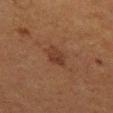biopsy status — total-body-photography surveillance lesion; no biopsy
imaging modality — ~15 mm crop, total-body skin-cancer survey
anatomic site — the left thigh
patient — female, approximately 50 years of age
tile lighting — cross-polarized illumination
automated metrics — about 6 CIELAB-L* units darker than the surrounding skin and a lesion-to-skin contrast of about 6 (normalized; higher = more distinct); border irregularity of about 2 on a 0–10 scale, a color-variation rating of about 3/10, and radial color variation of about 1